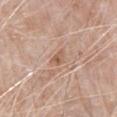{"patient": {"sex": "male", "age_approx": 80}, "image": {"source": "total-body photography crop", "field_of_view_mm": 15}, "lesion_size": {"long_diameter_mm_approx": 2.5}, "lighting": "white-light", "site": "chest"}